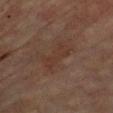Part of a total-body skin-imaging series; this lesion was reviewed on a skin check and was not flagged for biopsy.
From the chest.
Longest diameter approximately 4 mm.
Imaged with cross-polarized lighting.
The lesion-visualizer software estimated a footprint of about 6 mm² and two-axis asymmetry of about 0.4. The software also gave a border-irregularity rating of about 6.5/10 and radial color variation of about 0.5. It also reported a classifier nevus-likeness of about 0/100.
A male subject, in their mid- to late 70s.
A lesion tile, about 15 mm wide, cut from a 3D total-body photograph.